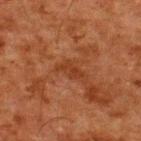Q: Was a biopsy performed?
A: total-body-photography surveillance lesion; no biopsy
Q: What is the imaging modality?
A: ~15 mm tile from a whole-body skin photo
Q: Who is the patient?
A: male, aged approximately 60
Q: What is the anatomic site?
A: the upper back